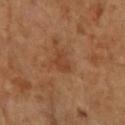This lesion was catalogued during total-body skin photography and was not selected for biopsy. Automated image analysis of the tile measured a border-irregularity rating of about 3/10, a color-variation rating of about 1.5/10, and radial color variation of about 0.5. And it measured a classifier nevus-likeness of about 0/100. A close-up tile cropped from a whole-body skin photograph, about 15 mm across. The subject is a female approximately 70 years of age. From the right forearm. The lesion's longest dimension is about 2.5 mm. Captured under cross-polarized illumination.